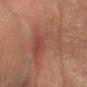biopsy_status: not biopsied; imaged during a skin examination
image:
  source: total-body photography crop
  field_of_view_mm: 15
lesion_size:
  long_diameter_mm_approx: 9.0
site: head or neck
lighting: cross-polarized
patient:
  sex: female
  age_approx: 70